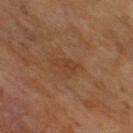biopsy_status: not biopsied; imaged during a skin examination
automated_metrics:
  vs_skin_darker_L: 5.0
  lesion_detection_confidence_0_100: 100
image:
  source: total-body photography crop
  field_of_view_mm: 15
lighting: cross-polarized
lesion_size:
  long_diameter_mm_approx: 3.5
patient:
  sex: male
  age_approx: 65